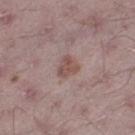The lesion was tiled from a total-body skin photograph and was not biopsied. A 15 mm close-up extracted from a 3D total-body photography capture. Imaged with white-light lighting. The subject is a male aged around 50. Measured at roughly 2.5 mm in maximum diameter. On the left thigh.